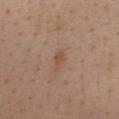The lesion was tiled from a total-body skin photograph and was not biopsied. A 15 mm close-up extracted from a 3D total-body photography capture. A female subject, aged 48 to 52. On the front of the torso.